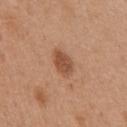This lesion was catalogued during total-body skin photography and was not selected for biopsy. Measured at roughly 3.5 mm in maximum diameter. A 15 mm crop from a total-body photograph taken for skin-cancer surveillance. A female subject, aged approximately 40. Captured under white-light illumination. Located on the chest.